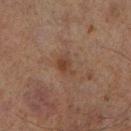follow-up=imaged on a skin check; not biopsied.